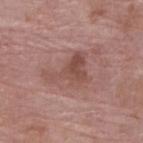Case summary:
- follow-up · catalogued during a skin exam; not biopsied
- image · ~15 mm crop, total-body skin-cancer survey
- lesion size · ~5.5 mm (longest diameter)
- site · the right lower leg
- patient · female, aged 68–72
- illumination · white-light